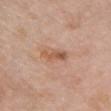notes = imaged on a skin check; not biopsied | patient = female, aged around 60 | imaging modality = ~15 mm tile from a whole-body skin photo | lesion size = ≈3.5 mm | site = the chest | tile lighting = white-light illumination.